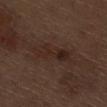Recorded during total-body skin imaging; not selected for excision or biopsy. The lesion's longest dimension is about 6 mm. The subject is a male roughly 70 years of age. Located on the right thigh. A region of skin cropped from a whole-body photographic capture, roughly 15 mm wide.